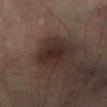No biopsy was performed on this lesion — it was imaged during a full skin examination and was not determined to be concerning. The recorded lesion diameter is about 4 mm. The lesion is located on the left lower leg. The patient is a male aged around 65. A 15 mm close-up extracted from a 3D total-body photography capture.